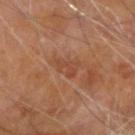The lesion was photographed on a routine skin check and not biopsied; there is no pathology result.